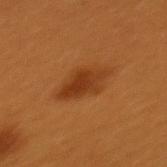Impression: Recorded during total-body skin imaging; not selected for excision or biopsy. Context: On the upper back. Cropped from a whole-body photographic skin survey; the tile spans about 15 mm. Automated image analysis of the tile measured a footprint of about 9.5 mm², an eccentricity of roughly 0.85, and a symmetry-axis asymmetry near 0.2. The software also gave radial color variation of about 1. The analysis additionally found a classifier nevus-likeness of about 95/100 and a lesion-detection confidence of about 100/100. A female patient, roughly 30 years of age. Captured under cross-polarized illumination.